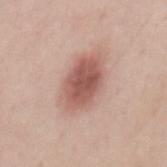notes: total-body-photography surveillance lesion; no biopsy | patient: male, about 25 years old | image: ~15 mm tile from a whole-body skin photo | body site: the back | size: ≈6 mm | illumination: white-light illumination | automated metrics: an area of roughly 16 mm², an outline eccentricity of about 0.8 (0 = round, 1 = elongated), and two-axis asymmetry of about 0.15; an average lesion color of about L≈56 a*≈23 b*≈25 (CIELAB), roughly 14 lightness units darker than nearby skin, and a normalized lesion–skin contrast near 9; lesion-presence confidence of about 100/100.